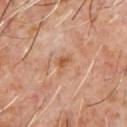Impression:
No biopsy was performed on this lesion — it was imaged during a full skin examination and was not determined to be concerning.
Context:
A region of skin cropped from a whole-body photographic capture, roughly 15 mm wide. Imaged with cross-polarized lighting. Longest diameter approximately 2.5 mm. A male subject, roughly 60 years of age. The total-body-photography lesion software estimated an area of roughly 3.5 mm², an eccentricity of roughly 0.7, and a shape-asymmetry score of about 0.4 (0 = symmetric). It also reported a border-irregularity index near 4/10, a within-lesion color-variation index near 1.5/10, and a peripheral color-asymmetry measure near 0.5. From the chest.